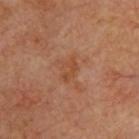Q: Was a biopsy performed?
A: no biopsy performed (imaged during a skin exam)
Q: What are the patient's age and sex?
A: aged 63 to 67
Q: How was this image acquired?
A: ~15 mm tile from a whole-body skin photo
Q: Lesion location?
A: the upper back
Q: What lighting was used for the tile?
A: cross-polarized illumination
Q: How large is the lesion?
A: ≈3 mm